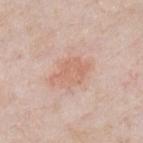Notes:
– notes: no biopsy performed (imaged during a skin exam)
– TBP lesion metrics: a lesion area of about 8 mm², an eccentricity of roughly 0.75, and a shape-asymmetry score of about 0.4 (0 = symmetric); a mean CIELAB color near L≈64 a*≈22 b*≈29, about 7 CIELAB-L* units darker than the surrounding skin, and a normalized border contrast of about 5.5; a nevus-likeness score of about 85/100 and a detector confidence of about 100 out of 100 that the crop contains a lesion
– imaging modality: ~15 mm crop, total-body skin-cancer survey
– lighting: white-light
– lesion diameter: ~4.5 mm (longest diameter)
– patient: male, about 50 years old
– body site: the chest